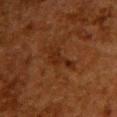Notes:
- follow-up — catalogued during a skin exam; not biopsied
- illumination — cross-polarized
- diameter — about 4.5 mm
- site — the upper back
- imaging modality — total-body-photography crop, ~15 mm field of view
- patient — female, aged 48 to 52